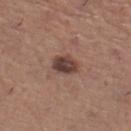Impression: Part of a total-body skin-imaging series; this lesion was reviewed on a skin check and was not flagged for biopsy. Clinical summary: From the right thigh. Captured under white-light illumination. Measured at roughly 3 mm in maximum diameter. A roughly 15 mm field-of-view crop from a total-body skin photograph. A female subject approximately 65 years of age.